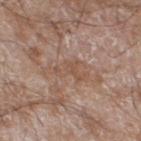<lesion>
<biopsy_status>not biopsied; imaged during a skin examination</biopsy_status>
<image>
  <source>total-body photography crop</source>
  <field_of_view_mm>15</field_of_view_mm>
</image>
<automated_metrics>
  <eccentricity>0.8</eccentricity>
  <shape_asymmetry>0.6</shape_asymmetry>
  <cielab_L>52</cielab_L>
  <cielab_a>18</cielab_a>
  <cielab_b>28</cielab_b>
  <vs_skin_darker_L>6.0</vs_skin_darker_L>
  <vs_skin_contrast_norm>5.0</vs_skin_contrast_norm>
  <border_irregularity_0_10>8.5</border_irregularity_0_10>
  <color_variation_0_10>1.0</color_variation_0_10>
  <peripheral_color_asymmetry>0.5</peripheral_color_asymmetry>
</automated_metrics>
<patient>
  <sex>male</sex>
  <age_approx>60</age_approx>
</patient>
<site>left lower leg</site>
<lesion_size>
  <long_diameter_mm_approx>4.0</long_diameter_mm_approx>
</lesion_size>
</lesion>